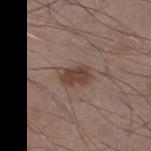Q: Was a biopsy performed?
A: imaged on a skin check; not biopsied
Q: Lesion size?
A: about 3.5 mm
Q: What are the patient's age and sex?
A: male, about 60 years old
Q: What is the imaging modality?
A: ~15 mm tile from a whole-body skin photo
Q: What did automated image analysis measure?
A: border irregularity of about 3 on a 0–10 scale and peripheral color asymmetry of about 0.5; an automated nevus-likeness rating near 90 out of 100 and a detector confidence of about 100 out of 100 that the crop contains a lesion
Q: Where on the body is the lesion?
A: the left thigh